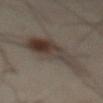Image and clinical context:
Imaged with cross-polarized lighting. A male subject in their mid-50s. From the abdomen. This image is a 15 mm lesion crop taken from a total-body photograph.